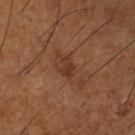Impression:
Captured during whole-body skin photography for melanoma surveillance; the lesion was not biopsied.
Background:
The subject is a male in their mid-60s. The total-body-photography lesion software estimated an area of roughly 3.5 mm², an eccentricity of roughly 0.7, and two-axis asymmetry of about 0.3. The analysis additionally found internal color variation of about 1.5 on a 0–10 scale. It also reported a detector confidence of about 100 out of 100 that the crop contains a lesion. A close-up tile cropped from a whole-body skin photograph, about 15 mm across. The lesion is located on the leg. Captured under cross-polarized illumination.